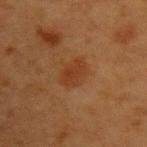Notes:
- biopsy status — total-body-photography surveillance lesion; no biopsy
- illumination — cross-polarized
- diameter — about 3.5 mm
- patient — female, aged approximately 40
- site — the left upper arm
- acquisition — total-body-photography crop, ~15 mm field of view